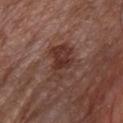No biopsy was performed on this lesion — it was imaged during a full skin examination and was not determined to be concerning.
Imaged with white-light lighting.
The lesion is located on the front of the torso.
A male patient, in their 80s.
The recorded lesion diameter is about 5 mm.
A lesion tile, about 15 mm wide, cut from a 3D total-body photograph.